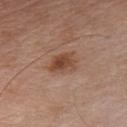| key | value |
|---|---|
| biopsy status | total-body-photography surveillance lesion; no biopsy |
| body site | the chest |
| subject | male, aged approximately 55 |
| lesion size | ≈3.5 mm |
| automated lesion analysis | a lesion–skin lightness drop of about 10 and a normalized lesion–skin contrast near 8; a border-irregularity index near 2.5/10, a within-lesion color-variation index near 4.5/10, and radial color variation of about 1.5; an automated nevus-likeness rating near 85 out of 100 and a lesion-detection confidence of about 100/100 |
| image source | total-body-photography crop, ~15 mm field of view |
| lighting | white-light |